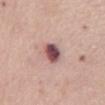biopsy_status: not biopsied; imaged during a skin examination
patient:
  sex: female
  age_approx: 65
lesion_size:
  long_diameter_mm_approx: 3.5
image:
  source: total-body photography crop
  field_of_view_mm: 15
site: abdomen
lighting: white-light
automated_metrics:
  border_irregularity_0_10: 2.5
  peripheral_color_asymmetry: 1.5